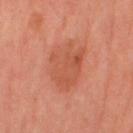follow-up = total-body-photography surveillance lesion; no biopsy | patient = female, about 50 years old | image = 15 mm crop, total-body photography | illumination = cross-polarized illumination | lesion diameter = ~5.5 mm (longest diameter) | location = the right thigh.